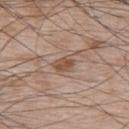Imaged during a routine full-body skin examination; the lesion was not biopsied and no histopathology is available. The lesion's longest dimension is about 2.5 mm. A male patient, aged around 65. On the upper back. An algorithmic analysis of the crop reported a border-irregularity index near 2.5/10, internal color variation of about 2.5 on a 0–10 scale, and peripheral color asymmetry of about 1. The software also gave a nevus-likeness score of about 30/100 and a lesion-detection confidence of about 100/100. Captured under white-light illumination. A 15 mm close-up extracted from a 3D total-body photography capture.